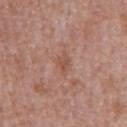A male subject roughly 65 years of age.
Automated tile analysis of the lesion measured a lesion area of about 3 mm², a shape eccentricity near 0.75, and a symmetry-axis asymmetry near 0.45. The analysis additionally found roughly 7 lightness units darker than nearby skin and a normalized lesion–skin contrast near 6. The analysis additionally found a classifier nevus-likeness of about 0/100 and a lesion-detection confidence of about 100/100.
Cropped from a whole-body photographic skin survey; the tile spans about 15 mm.
The recorded lesion diameter is about 2.5 mm.
The lesion is on the abdomen.